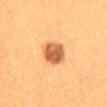follow-up — catalogued during a skin exam; not biopsied | imaging modality — ~15 mm tile from a whole-body skin photo | automated metrics — a footprint of about 9 mm², an outline eccentricity of about 0.65 (0 = round, 1 = elongated), and two-axis asymmetry of about 0.1; a mean CIELAB color near L≈50 a*≈22 b*≈36, roughly 14 lightness units darker than nearby skin, and a normalized border contrast of about 10; a border-irregularity rating of about 1/10, a within-lesion color-variation index near 4/10, and peripheral color asymmetry of about 1.5 | lesion size — about 4 mm | subject — female, approximately 40 years of age | tile lighting — cross-polarized | site — the lower back.